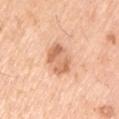This lesion was catalogued during total-body skin photography and was not selected for biopsy.
Automated tile analysis of the lesion measured an area of roughly 9 mm² and a shape eccentricity near 0.6.
About 3.5 mm across.
This image is a 15 mm lesion crop taken from a total-body photograph.
A male subject, in their mid-50s.
The lesion is located on the left upper arm.